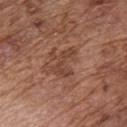Assessment: Part of a total-body skin-imaging series; this lesion was reviewed on a skin check and was not flagged for biopsy. Image and clinical context: A lesion tile, about 15 mm wide, cut from a 3D total-body photograph. Automated image analysis of the tile measured a footprint of about 5.5 mm² and an outline eccentricity of about 0.85 (0 = round, 1 = elongated). The software also gave a mean CIELAB color near L≈43 a*≈22 b*≈28 and about 8 CIELAB-L* units darker than the surrounding skin. It also reported a border-irregularity index near 8/10 and internal color variation of about 1.5 on a 0–10 scale. The analysis additionally found a nevus-likeness score of about 0/100 and a lesion-detection confidence of about 100/100. A male patient roughly 75 years of age. The lesion's longest dimension is about 4 mm. The lesion is on the chest.